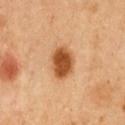Background:
A 15 mm close-up tile from a total-body photography series done for melanoma screening. A male subject, roughly 50 years of age. From the chest.